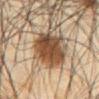This lesion was catalogued during total-body skin photography and was not selected for biopsy. Measured at roughly 6.5 mm in maximum diameter. Cropped from a total-body skin-imaging series; the visible field is about 15 mm. Captured under cross-polarized illumination. The lesion is on the abdomen. An algorithmic analysis of the crop reported a border-irregularity rating of about 3/10 and a color-variation rating of about 5/10. The analysis additionally found a classifier nevus-likeness of about 100/100 and lesion-presence confidence of about 100/100. A male patient, about 65 years old.